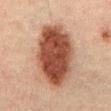<record>
  <biopsy_status>not biopsied; imaged during a skin examination</biopsy_status>
  <image>
    <source>total-body photography crop</source>
    <field_of_view_mm>15</field_of_view_mm>
  </image>
  <automated_metrics>
    <area_mm2_approx>36.0</area_mm2_approx>
    <eccentricity>0.8</eccentricity>
    <shape_asymmetry>0.1</shape_asymmetry>
  </automated_metrics>
  <lesion_size>
    <long_diameter_mm_approx>9.0</long_diameter_mm_approx>
  </lesion_size>
  <patient>
    <sex>male</sex>
    <age_approx>50</age_approx>
  </patient>
  <site>mid back</site>
</record>